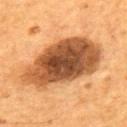workup = no biopsy performed (imaged during a skin exam) | subject = male, roughly 55 years of age | body site = the back | imaging modality = ~15 mm crop, total-body skin-cancer survey.